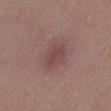Clinical impression: Imaged during a routine full-body skin examination; the lesion was not biopsied and no histopathology is available. Context: This is a white-light tile. The patient is a female roughly 50 years of age. Cropped from a total-body skin-imaging series; the visible field is about 15 mm. The lesion is located on the back. The lesion's longest dimension is about 4 mm. Automated tile analysis of the lesion measured a lesion color around L≈45 a*≈20 b*≈18 in CIELAB, about 7 CIELAB-L* units darker than the surrounding skin, and a lesion-to-skin contrast of about 6 (normalized; higher = more distinct). The analysis additionally found a border-irregularity rating of about 2.5/10, internal color variation of about 2.5 on a 0–10 scale, and a peripheral color-asymmetry measure near 0.5.